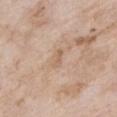Part of a total-body skin-imaging series; this lesion was reviewed on a skin check and was not flagged for biopsy.
The subject is a female in their mid-70s.
The tile uses white-light illumination.
Measured at roughly 2.5 mm in maximum diameter.
From the chest.
A region of skin cropped from a whole-body photographic capture, roughly 15 mm wide.
Automated image analysis of the tile measured a footprint of about 2.5 mm², an outline eccentricity of about 0.9 (0 = round, 1 = elongated), and a symmetry-axis asymmetry near 0.3. The analysis additionally found a lesion color around L≈61 a*≈17 b*≈31 in CIELAB, a lesion–skin lightness drop of about 7, and a lesion-to-skin contrast of about 5 (normalized; higher = more distinct). It also reported a border-irregularity rating of about 3/10 and a within-lesion color-variation index near 0/10.